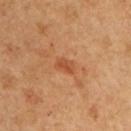The lesion was photographed on a routine skin check and not biopsied; there is no pathology result.
From the front of the torso.
A region of skin cropped from a whole-body photographic capture, roughly 15 mm wide.
A male patient, roughly 50 years of age.